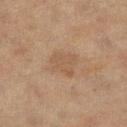Imaged during a routine full-body skin examination; the lesion was not biopsied and no histopathology is available.
This image is a 15 mm lesion crop taken from a total-body photograph.
From the right lower leg.
A female subject, roughly 80 years of age.